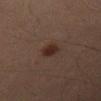workup — catalogued during a skin exam; not biopsied
lighting — cross-polarized
imaging modality — ~15 mm crop, total-body skin-cancer survey
patient — male, aged around 70
anatomic site — the left thigh
TBP lesion metrics — a footprint of about 4 mm², a shape eccentricity near 0.65, and two-axis asymmetry of about 0.25; a lesion color around L≈21 a*≈14 b*≈19 in CIELAB and roughly 7 lightness units darker than nearby skin; a border-irregularity rating of about 2/10 and peripheral color asymmetry of about 0.5
diameter — ~2.5 mm (longest diameter)